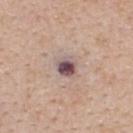follow-up: no biopsy performed (imaged during a skin exam)
illumination: white-light illumination
patient: female, aged 43 to 47
imaging modality: total-body-photography crop, ~15 mm field of view
location: the upper back
size: about 2.5 mm
image-analysis metrics: a footprint of about 5.5 mm²; about 16 CIELAB-L* units darker than the surrounding skin